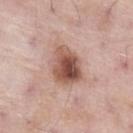| field | value |
|---|---|
| follow-up | total-body-photography surveillance lesion; no biopsy |
| image | ~15 mm tile from a whole-body skin photo |
| subject | male, approximately 55 years of age |
| site | the right thigh |